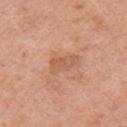workup=no biopsy performed (imaged during a skin exam); anatomic site=the right upper arm; patient=female, roughly 50 years of age; acquisition=total-body-photography crop, ~15 mm field of view; size=≈4 mm; illumination=white-light.